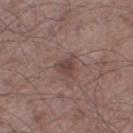Acquisition and patient details: Approximately 3 mm at its widest. On the right thigh. A 15 mm crop from a total-body photograph taken for skin-cancer surveillance. The tile uses white-light illumination. A male subject in their mid- to late 50s.